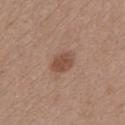subject = female, aged 33 to 37
anatomic site = the chest
acquisition = 15 mm crop, total-body photography